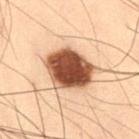workup = imaged on a skin check; not biopsied
location = the leg
tile lighting = cross-polarized illumination
imaging modality = ~15 mm tile from a whole-body skin photo
patient = male, about 55 years old